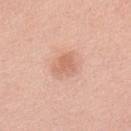This image is a 15 mm lesion crop taken from a total-body photograph. The patient is a male aged approximately 55. The total-body-photography lesion software estimated an area of roughly 5.5 mm², a shape eccentricity near 0.65, and a symmetry-axis asymmetry near 0.35. The software also gave a lesion color around L≈65 a*≈24 b*≈31 in CIELAB and a lesion–skin lightness drop of about 9. The analysis additionally found a peripheral color-asymmetry measure near 0.5. On the back. Captured under white-light illumination.